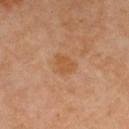• follow-up · no biopsy performed (imaged during a skin exam)
• patient · female, aged around 50
• body site · the right lower leg
• TBP lesion metrics · a lesion area of about 4 mm² and two-axis asymmetry of about 0.3; a lesion-to-skin contrast of about 6.5 (normalized; higher = more distinct); a border-irregularity index near 2.5/10, internal color variation of about 1 on a 0–10 scale, and a peripheral color-asymmetry measure near 0.5
• image · ~15 mm tile from a whole-body skin photo
• illumination · cross-polarized illumination
• diameter · ~2.5 mm (longest diameter)